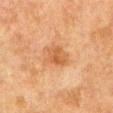{"biopsy_status": "not biopsied; imaged during a skin examination", "lesion_size": {"long_diameter_mm_approx": 3.5}, "patient": {"sex": "male", "age_approx": 80}, "lighting": "cross-polarized", "site": "abdomen", "image": {"source": "total-body photography crop", "field_of_view_mm": 15}, "automated_metrics": {"area_mm2_approx": 6.5, "border_irregularity_0_10": 1.5, "color_variation_0_10": 3.0, "peripheral_color_asymmetry": 1.0, "nevus_likeness_0_100": 15, "lesion_detection_confidence_0_100": 100}}